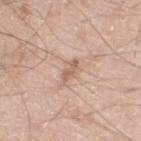notes = imaged on a skin check; not biopsied
patient = male, approximately 60 years of age
lighting = white-light illumination
acquisition = ~15 mm tile from a whole-body skin photo
site = the left lower leg
size = ≈3 mm
automated metrics = a footprint of about 3 mm²; an average lesion color of about L≈61 a*≈19 b*≈29 (CIELAB), roughly 10 lightness units darker than nearby skin, and a normalized lesion–skin contrast near 6.5; border irregularity of about 3.5 on a 0–10 scale and a peripheral color-asymmetry measure near 0